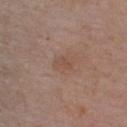This lesion was catalogued during total-body skin photography and was not selected for biopsy. A 15 mm close-up extracted from a 3D total-body photography capture. The total-body-photography lesion software estimated an average lesion color of about L≈50 a*≈18 b*≈27 (CIELAB) and about 5 CIELAB-L* units darker than the surrounding skin. A male patient aged around 70. The lesion is located on the chest.